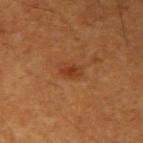Image and clinical context:
A male patient aged approximately 60. The total-body-photography lesion software estimated a lesion color around L≈35 a*≈24 b*≈33 in CIELAB, about 7 CIELAB-L* units darker than the surrounding skin, and a normalized lesion–skin contrast near 6.5. The software also gave a border-irregularity index near 2.5/10. The analysis additionally found a nevus-likeness score of about 50/100 and a lesion-detection confidence of about 100/100. A close-up tile cropped from a whole-body skin photograph, about 15 mm across. The tile uses cross-polarized illumination. Longest diameter approximately 2.5 mm. Located on the arm.